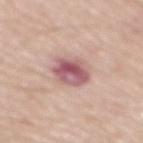Recorded during total-body skin imaging; not selected for excision or biopsy. A male patient approximately 70 years of age. The lesion is located on the mid back. Imaged with white-light lighting. An algorithmic analysis of the crop reported an average lesion color of about L≈58 a*≈24 b*≈19 (CIELAB), about 14 CIELAB-L* units darker than the surrounding skin, and a lesion-to-skin contrast of about 9.5 (normalized; higher = more distinct). And it measured a border-irregularity index near 1.5/10 and a within-lesion color-variation index near 8.5/10. Cropped from a total-body skin-imaging series; the visible field is about 15 mm. Measured at roughly 4 mm in maximum diameter.